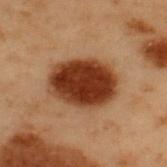Clinical impression: Recorded during total-body skin imaging; not selected for excision or biopsy. Acquisition and patient details: About 6.5 mm across. The patient is a male approximately 55 years of age. A 15 mm crop from a total-body photograph taken for skin-cancer surveillance. The lesion is located on the back. The lesion-visualizer software estimated an area of roughly 26 mm², an outline eccentricity of about 0.7 (0 = round, 1 = elongated), and a symmetry-axis asymmetry near 0.1. The software also gave a lesion color around L≈30 a*≈21 b*≈29 in CIELAB, roughly 16 lightness units darker than nearby skin, and a lesion-to-skin contrast of about 14.5 (normalized; higher = more distinct). It also reported a within-lesion color-variation index near 5/10 and a peripheral color-asymmetry measure near 1.5.